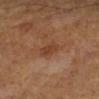<lesion>
  <patient>
    <sex>male</sex>
    <age_approx>60</age_approx>
  </patient>
  <lesion_size>
    <long_diameter_mm_approx>2.5</long_diameter_mm_approx>
  </lesion_size>
  <site>right lower leg</site>
  <image>
    <source>total-body photography crop</source>
    <field_of_view_mm>15</field_of_view_mm>
  </image>
  <lighting>cross-polarized</lighting>
</lesion>